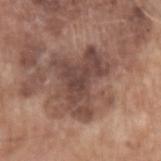This lesion was catalogued during total-body skin photography and was not selected for biopsy. Imaged with white-light lighting. A 15 mm close-up tile from a total-body photography series done for melanoma screening. An algorithmic analysis of the crop reported an area of roughly 25 mm² and an eccentricity of roughly 0.65. It also reported a peripheral color-asymmetry measure near 1.5. A male subject, in their mid-70s. The lesion is located on the left upper arm.